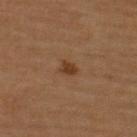Impression: Part of a total-body skin-imaging series; this lesion was reviewed on a skin check and was not flagged for biopsy. Image and clinical context: A male subject, in their mid-60s. This is a cross-polarized tile. The lesion is on the upper back. Approximately 2 mm at its widest. A roughly 15 mm field-of-view crop from a total-body skin photograph. The total-body-photography lesion software estimated border irregularity of about 3 on a 0–10 scale and peripheral color asymmetry of about 0.